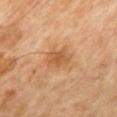<lesion>
  <biopsy_status>not biopsied; imaged during a skin examination</biopsy_status>
  <lighting>cross-polarized</lighting>
  <lesion_size>
    <long_diameter_mm_approx>4.0</long_diameter_mm_approx>
  </lesion_size>
  <site>mid back</site>
  <automated_metrics>
    <area_mm2_approx>7.0</area_mm2_approx>
    <eccentricity>0.75</eccentricity>
    <shape_asymmetry>0.25</shape_asymmetry>
    <cielab_L>56</cielab_L>
    <cielab_a>23</cielab_a>
    <cielab_b>39</cielab_b>
    <vs_skin_contrast_norm>6.5</vs_skin_contrast_norm>
    <border_irregularity_0_10>2.5</border_irregularity_0_10>
    <color_variation_0_10>3.5</color_variation_0_10>
    <peripheral_color_asymmetry>1.0</peripheral_color_asymmetry>
  </automated_metrics>
  <image>
    <source>total-body photography crop</source>
    <field_of_view_mm>15</field_of_view_mm>
  </image>
  <patient>
    <age_approx>65</age_approx>
  </patient>
</lesion>